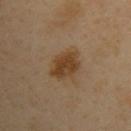Imaged during a routine full-body skin examination; the lesion was not biopsied and no histopathology is available. A close-up tile cropped from a whole-body skin photograph, about 15 mm across. A male patient, aged 38–42. This is a cross-polarized tile. Measured at roughly 4.5 mm in maximum diameter. Automated image analysis of the tile measured an area of roughly 10 mm², a shape eccentricity near 0.7, and a shape-asymmetry score of about 0.2 (0 = symmetric). And it measured a border-irregularity rating of about 2.5/10, a within-lesion color-variation index near 3/10, and a peripheral color-asymmetry measure near 1. It also reported a nevus-likeness score of about 100/100 and a detector confidence of about 100 out of 100 that the crop contains a lesion. On the left upper arm.